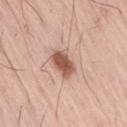workup — no biopsy performed (imaged during a skin exam); subject — male, in their mid-50s; image source — 15 mm crop, total-body photography; site — the right thigh.